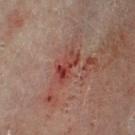Notes:
• follow-up — total-body-photography surveillance lesion; no biopsy
• acquisition — total-body-photography crop, ~15 mm field of view
• subject — female, aged approximately 80
• automated metrics — a mean CIELAB color near L≈37 a*≈27 b*≈24, a lesion–skin lightness drop of about 9, and a lesion-to-skin contrast of about 8 (normalized; higher = more distinct)
• lesion size — about 4 mm
• body site — the right leg